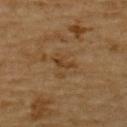Clinical impression: Part of a total-body skin-imaging series; this lesion was reviewed on a skin check and was not flagged for biopsy. Background: Cropped from a whole-body photographic skin survey; the tile spans about 15 mm. This is a cross-polarized tile. The total-body-photography lesion software estimated a lesion color around L≈33 a*≈16 b*≈30 in CIELAB and a normalized border contrast of about 6.5. And it measured border irregularity of about 4.5 on a 0–10 scale, a color-variation rating of about 0.5/10, and peripheral color asymmetry of about 0. A male patient aged 83 or older. Located on the upper back. The lesion's longest dimension is about 2.5 mm.